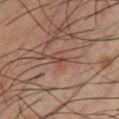A male patient, aged approximately 40. A region of skin cropped from a whole-body photographic capture, roughly 15 mm wide. The lesion is located on the chest. The total-body-photography lesion software estimated a lesion area of about 2 mm² and a shape-asymmetry score of about 0.55 (0 = symmetric). The software also gave an automated nevus-likeness rating near 0 out of 100 and a detector confidence of about 85 out of 100 that the crop contains a lesion. Longest diameter approximately 2.5 mm. Captured under cross-polarized illumination.